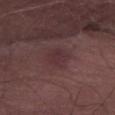Captured during whole-body skin photography for melanoma surveillance; the lesion was not biopsied. A roughly 15 mm field-of-view crop from a total-body skin photograph. An algorithmic analysis of the crop reported an outline eccentricity of about 0.55 (0 = round, 1 = elongated). It also reported a lesion–skin lightness drop of about 5. The analysis additionally found a nevus-likeness score of about 0/100 and lesion-presence confidence of about 90/100. A male subject, aged 58 to 62. Located on the right thigh. Approximately 3 mm at its widest.